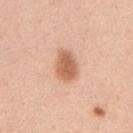On the front of the torso. The lesion's longest dimension is about 3.5 mm. Cropped from a total-body skin-imaging series; the visible field is about 15 mm. The patient is a male in their 50s.